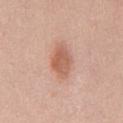<case>
<biopsy_status>not biopsied; imaged during a skin examination</biopsy_status>
<image>
  <source>total-body photography crop</source>
  <field_of_view_mm>15</field_of_view_mm>
</image>
<automated_metrics>
  <eccentricity>0.8</eccentricity>
  <shape_asymmetry>0.2</shape_asymmetry>
  <vs_skin_darker_L>11.0</vs_skin_darker_L>
  <vs_skin_contrast_norm>7.0</vs_skin_contrast_norm>
  <border_irregularity_0_10>2.0</border_irregularity_0_10>
  <color_variation_0_10>3.0</color_variation_0_10>
  <nevus_likeness_0_100>80</nevus_likeness_0_100>
  <lesion_detection_confidence_0_100>100</lesion_detection_confidence_0_100>
</automated_metrics>
<site>abdomen</site>
<patient>
  <sex>male</sex>
  <age_approx>35</age_approx>
</patient>
<lesion_size>
  <long_diameter_mm_approx>4.5</long_diameter_mm_approx>
</lesion_size>
<lighting>white-light</lighting>
</case>